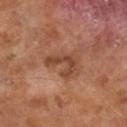<tbp_lesion>
  <biopsy_status>not biopsied; imaged during a skin examination</biopsy_status>
  <lesion_size>
    <long_diameter_mm_approx>4.0</long_diameter_mm_approx>
  </lesion_size>
  <automated_metrics>
    <border_irregularity_0_10>8.0</border_irregularity_0_10>
    <color_variation_0_10>2.0</color_variation_0_10>
    <peripheral_color_asymmetry>0.5</peripheral_color_asymmetry>
    <nevus_likeness_0_100>0</nevus_likeness_0_100>
    <lesion_detection_confidence_0_100>100</lesion_detection_confidence_0_100>
  </automated_metrics>
  <patient>
    <sex>male</sex>
    <age_approx>65</age_approx>
  </patient>
  <lighting>cross-polarized</lighting>
  <image>
    <source>total-body photography crop</source>
    <field_of_view_mm>15</field_of_view_mm>
  </image>
</tbp_lesion>